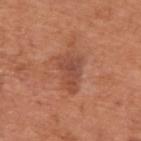Q: Is there a histopathology result?
A: catalogued during a skin exam; not biopsied
Q: What kind of image is this?
A: 15 mm crop, total-body photography
Q: Lesion location?
A: the upper back
Q: Who is the patient?
A: male, approximately 65 years of age